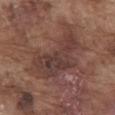A male subject, approximately 75 years of age. A region of skin cropped from a whole-body photographic capture, roughly 15 mm wide. From the front of the torso.